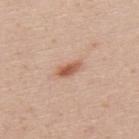Impression: No biopsy was performed on this lesion — it was imaged during a full skin examination and was not determined to be concerning. Clinical summary: The lesion's longest dimension is about 3 mm. The patient is a male about 35 years old. Imaged with white-light lighting. On the upper back. A roughly 15 mm field-of-view crop from a total-body skin photograph.